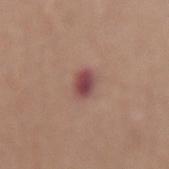Part of a total-body skin-imaging series; this lesion was reviewed on a skin check and was not flagged for biopsy. Located on the mid back. A male patient, aged 73 to 77. A 15 mm close-up tile from a total-body photography series done for melanoma screening.